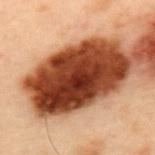| field | value |
|---|---|
| biopsy status | imaged on a skin check; not biopsied |
| patient | male, aged 53–57 |
| lesion diameter | ≈10 mm |
| body site | the upper back |
| automated lesion analysis | a footprint of about 49 mm², a shape eccentricity near 0.8, and two-axis asymmetry of about 0.1; a border-irregularity index near 1.5/10, internal color variation of about 7.5 on a 0–10 scale, and radial color variation of about 2.5 |
| image source | 15 mm crop, total-body photography |